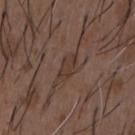Part of a total-body skin-imaging series; this lesion was reviewed on a skin check and was not flagged for biopsy. A male subject roughly 50 years of age. Cropped from a whole-body photographic skin survey; the tile spans about 15 mm. On the chest.